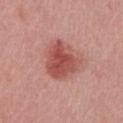Notes:
- workup · catalogued during a skin exam; not biopsied
- image source · total-body-photography crop, ~15 mm field of view
- illumination · white-light
- automated lesion analysis · a shape eccentricity near 0.6 and a symmetry-axis asymmetry near 0.3; a lesion–skin lightness drop of about 12 and a lesion-to-skin contrast of about 8.5 (normalized; higher = more distinct); a border-irregularity rating of about 3/10, a color-variation rating of about 4/10, and radial color variation of about 1
- site · the front of the torso
- subject · male, aged approximately 50
- lesion diameter · about 5 mm